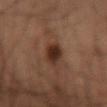Part of a total-body skin-imaging series; this lesion was reviewed on a skin check and was not flagged for biopsy. A close-up tile cropped from a whole-body skin photograph, about 15 mm across. A male patient, roughly 55 years of age. Located on the right forearm. Captured under cross-polarized illumination. The recorded lesion diameter is about 3 mm.